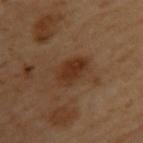This lesion was catalogued during total-body skin photography and was not selected for biopsy. Located on the upper back. A female subject aged approximately 60. A 15 mm close-up extracted from a 3D total-body photography capture.